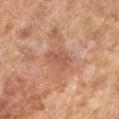Part of a total-body skin-imaging series; this lesion was reviewed on a skin check and was not flagged for biopsy.
The patient is a female aged 58 to 62.
Automated image analysis of the tile measured a border-irregularity rating of about 3/10, a within-lesion color-variation index near 2/10, and radial color variation of about 0.5.
From the left lower leg.
Cropped from a whole-body photographic skin survey; the tile spans about 15 mm.